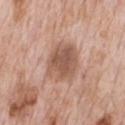Image and clinical context: A male subject, roughly 60 years of age. About 4.5 mm across. This image is a 15 mm lesion crop taken from a total-body photograph. Automated tile analysis of the lesion measured a lesion–skin lightness drop of about 12 and a lesion-to-skin contrast of about 8 (normalized; higher = more distinct). The software also gave internal color variation of about 4 on a 0–10 scale and peripheral color asymmetry of about 1.5. It also reported an automated nevus-likeness rating near 10 out of 100. This is a white-light tile. Located on the chest.